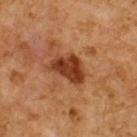Clinical impression:
This lesion was catalogued during total-body skin photography and was not selected for biopsy.
Context:
Located on the upper back. This image is a 15 mm lesion crop taken from a total-body photograph. Captured under cross-polarized illumination. A male patient roughly 60 years of age. About 4.5 mm across. An algorithmic analysis of the crop reported a lesion area of about 10 mm². And it measured a mean CIELAB color near L≈35 a*≈25 b*≈33 and roughly 13 lightness units darker than nearby skin. The analysis additionally found an automated nevus-likeness rating near 85 out of 100 and lesion-presence confidence of about 100/100.